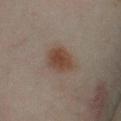The lesion was tiled from a total-body skin photograph and was not biopsied. About 4 mm across. The subject is a female in their mid-30s. On the left lower leg. The tile uses cross-polarized illumination. Cropped from a whole-body photographic skin survey; the tile spans about 15 mm.